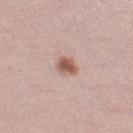The lesion was photographed on a routine skin check and not biopsied; there is no pathology result.
The subject is a female aged around 20.
An algorithmic analysis of the crop reported a border-irregularity index near 2.5/10, internal color variation of about 3.5 on a 0–10 scale, and peripheral color asymmetry of about 1. And it measured a nevus-likeness score of about 95/100 and lesion-presence confidence of about 100/100.
Imaged with white-light lighting.
Located on the left thigh.
A close-up tile cropped from a whole-body skin photograph, about 15 mm across.